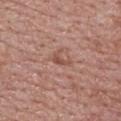workup: no biopsy performed (imaged during a skin exam)
image: total-body-photography crop, ~15 mm field of view
diameter: ~2.5 mm (longest diameter)
automated metrics: an eccentricity of roughly 0.9 and two-axis asymmetry of about 0.55; an average lesion color of about L≈50 a*≈22 b*≈26 (CIELAB), a lesion–skin lightness drop of about 8, and a normalized border contrast of about 6.5; an automated nevus-likeness rating near 0 out of 100 and lesion-presence confidence of about 100/100
site: the upper back
subject: male, roughly 70 years of age